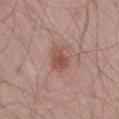Impression:
Imaged during a routine full-body skin examination; the lesion was not biopsied and no histopathology is available.
Background:
A male subject, roughly 50 years of age. Cropped from a total-body skin-imaging series; the visible field is about 15 mm. On the left thigh.